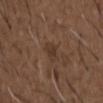<case>
  <biopsy_status>not biopsied; imaged during a skin examination</biopsy_status>
  <lighting>white-light</lighting>
  <automated_metrics>
    <area_mm2_approx>4.0</area_mm2_approx>
    <eccentricity>0.85</eccentricity>
    <shape_asymmetry>0.3</shape_asymmetry>
    <border_irregularity_0_10>3.0</border_irregularity_0_10>
    <color_variation_0_10>1.5</color_variation_0_10>
    <peripheral_color_asymmetry>0.5</peripheral_color_asymmetry>
    <nevus_likeness_0_100>0</nevus_likeness_0_100>
    <lesion_detection_confidence_0_100>100</lesion_detection_confidence_0_100>
  </automated_metrics>
  <image>
    <source>total-body photography crop</source>
    <field_of_view_mm>15</field_of_view_mm>
  </image>
  <patient>
    <sex>male</sex>
    <age_approx>50</age_approx>
  </patient>
  <site>front of the torso</site>
  <lesion_size>
    <long_diameter_mm_approx>3.0</long_diameter_mm_approx>
  </lesion_size>
</case>